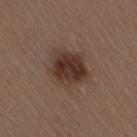notes = catalogued during a skin exam; not biopsied | body site = the lower back | lighting = white-light | image = total-body-photography crop, ~15 mm field of view | subject = male, roughly 70 years of age | diameter = about 5 mm.